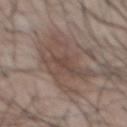Image and clinical context:
The subject is a male aged 53–57. Approximately 7 mm at its widest. The lesion-visualizer software estimated an average lesion color of about L≈47 a*≈14 b*≈22 (CIELAB), a lesion–skin lightness drop of about 8, and a normalized border contrast of about 6.5. The software also gave a border-irregularity index near 8/10. It also reported a classifier nevus-likeness of about 55/100. The lesion is located on the chest. This image is a 15 mm lesion crop taken from a total-body photograph. This is a white-light tile.